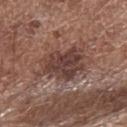Q: Was this lesion biopsied?
A: imaged on a skin check; not biopsied
Q: What is the anatomic site?
A: the arm
Q: What is the imaging modality?
A: ~15 mm tile from a whole-body skin photo
Q: Patient demographics?
A: male, aged around 70
Q: How was the tile lit?
A: white-light